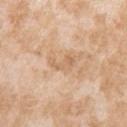Captured during whole-body skin photography for melanoma surveillance; the lesion was not biopsied. The recorded lesion diameter is about 3.5 mm. The total-body-photography lesion software estimated a lesion area of about 4.5 mm², a shape eccentricity near 0.9, and a symmetry-axis asymmetry near 0.45. The analysis additionally found a mean CIELAB color near L≈65 a*≈19 b*≈35 and roughly 8 lightness units darker than nearby skin. And it measured a color-variation rating of about 2/10 and peripheral color asymmetry of about 0.5. The analysis additionally found a classifier nevus-likeness of about 0/100. A female patient aged 23–27. Located on the arm. Captured under white-light illumination. This image is a 15 mm lesion crop taken from a total-body photograph.